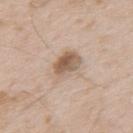Recorded during total-body skin imaging; not selected for excision or biopsy.
The lesion is located on the upper back.
This is a white-light tile.
Longest diameter approximately 4.5 mm.
A 15 mm crop from a total-body photograph taken for skin-cancer surveillance.
A male subject aged 63–67.